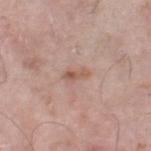Imaged during a routine full-body skin examination; the lesion was not biopsied and no histopathology is available. A male patient aged around 70. The recorded lesion diameter is about 2.5 mm. Cropped from a total-body skin-imaging series; the visible field is about 15 mm. Located on the right thigh.